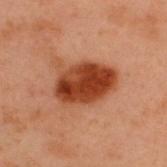Clinical impression: Recorded during total-body skin imaging; not selected for excision or biopsy.